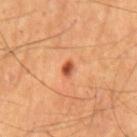follow-up: imaged on a skin check; not biopsied | image: ~15 mm tile from a whole-body skin photo | illumination: cross-polarized | subject: male, aged around 55 | TBP lesion metrics: a lesion area of about 2 mm², an eccentricity of roughly 0.75, and a shape-asymmetry score of about 0.3 (0 = symmetric); border irregularity of about 2 on a 0–10 scale and peripheral color asymmetry of about 0.5 | diameter: about 2 mm | anatomic site: the mid back.